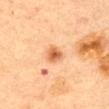image source: ~15 mm crop, total-body skin-cancer survey
location: the chest
subject: female, aged approximately 60
diameter: ≈2.5 mm
illumination: cross-polarized
image-analysis metrics: a footprint of about 4.5 mm², an eccentricity of roughly 0.65, and two-axis asymmetry of about 0.2; an average lesion color of about L≈57 a*≈24 b*≈38 (CIELAB), about 13 CIELAB-L* units darker than the surrounding skin, and a lesion-to-skin contrast of about 8.5 (normalized; higher = more distinct)